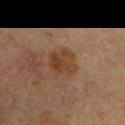subject: male, aged 58–62
location: the left upper arm
tile lighting: cross-polarized
lesion diameter: ≈3.5 mm
acquisition: ~15 mm tile from a whole-body skin photo